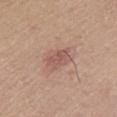  biopsy_status: not biopsied; imaged during a skin examination
  site: left upper arm
  image:
    source: total-body photography crop
    field_of_view_mm: 15
  patient:
    sex: female
    age_approx: 40